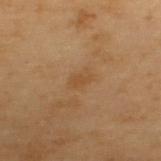The lesion was photographed on a routine skin check and not biopsied; there is no pathology result. Located on the upper back. The lesion's longest dimension is about 2.5 mm. Captured under cross-polarized illumination. An algorithmic analysis of the crop reported an eccentricity of roughly 0.75. The software also gave a mean CIELAB color near L≈39 a*≈15 b*≈30, roughly 4 lightness units darker than nearby skin, and a lesion-to-skin contrast of about 4.5 (normalized; higher = more distinct). The analysis additionally found a nevus-likeness score of about 0/100 and a detector confidence of about 100 out of 100 that the crop contains a lesion. A 15 mm crop from a total-body photograph taken for skin-cancer surveillance. The subject is a male about 55 years old.